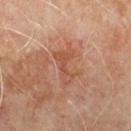Recorded during total-body skin imaging; not selected for excision or biopsy.
A male subject aged approximately 60.
Located on the back.
A lesion tile, about 15 mm wide, cut from a 3D total-body photograph.